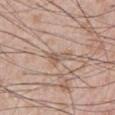Part of a total-body skin-imaging series; this lesion was reviewed on a skin check and was not flagged for biopsy. Longest diameter approximately 2.5 mm. The subject is a male aged 53 to 57. The lesion-visualizer software estimated a footprint of about 3 mm², a shape eccentricity near 0.85, and two-axis asymmetry of about 0.4. The software also gave an average lesion color of about L≈57 a*≈16 b*≈27 (CIELAB), about 8 CIELAB-L* units darker than the surrounding skin, and a normalized border contrast of about 6. On the abdomen. A roughly 15 mm field-of-view crop from a total-body skin photograph.